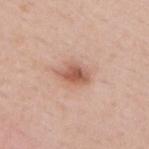Captured during whole-body skin photography for melanoma surveillance; the lesion was not biopsied. A close-up tile cropped from a whole-body skin photograph, about 15 mm across. The patient is a male aged approximately 50. The lesion's longest dimension is about 4 mm. Captured under white-light illumination. On the upper back. The total-body-photography lesion software estimated a lesion color around L≈58 a*≈23 b*≈30 in CIELAB and about 13 CIELAB-L* units darker than the surrounding skin.